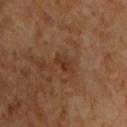<tbp_lesion>
<biopsy_status>not biopsied; imaged during a skin examination</biopsy_status>
<lesion_size>
  <long_diameter_mm_approx>3.0</long_diameter_mm_approx>
</lesion_size>
<image>
  <source>total-body photography crop</source>
  <field_of_view_mm>15</field_of_view_mm>
</image>
<patient>
  <sex>male</sex>
  <age_approx>65</age_approx>
</patient>
<lighting>cross-polarized</lighting>
</tbp_lesion>